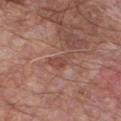<case>
<biopsy_status>not biopsied; imaged during a skin examination</biopsy_status>
<lighting>white-light</lighting>
<lesion_size>
  <long_diameter_mm_approx>3.5</long_diameter_mm_approx>
</lesion_size>
<image>
  <source>total-body photography crop</source>
  <field_of_view_mm>15</field_of_view_mm>
</image>
<automated_metrics>
  <area_mm2_approx>4.5</area_mm2_approx>
  <eccentricity>0.9</eccentricity>
  <shape_asymmetry>0.4</shape_asymmetry>
  <cielab_L>47</cielab_L>
  <cielab_a>23</cielab_a>
  <cielab_b>26</cielab_b>
  <vs_skin_darker_L>7.0</vs_skin_darker_L>
  <vs_skin_contrast_norm>6.0</vs_skin_contrast_norm>
  <border_irregularity_0_10>4.0</border_irregularity_0_10>
  <peripheral_color_asymmetry>0.5</peripheral_color_asymmetry>
  <nevus_likeness_0_100>0</nevus_likeness_0_100>
  <lesion_detection_confidence_0_100>95</lesion_detection_confidence_0_100>
</automated_metrics>
<patient>
  <sex>male</sex>
  <age_approx>65</age_approx>
</patient>
<site>chest</site>
</case>